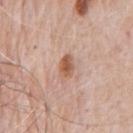The lesion was photographed on a routine skin check and not biopsied; there is no pathology result.
From the chest.
A male subject aged approximately 80.
This image is a 15 mm lesion crop taken from a total-body photograph.
This is a white-light tile.
Longest diameter approximately 2.5 mm.